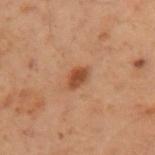Notes:
* biopsy status: imaged on a skin check; not biopsied
* image: ~15 mm tile from a whole-body skin photo
* illumination: cross-polarized illumination
* patient: female, about 55 years old
* anatomic site: the arm
* lesion diameter: ~2.5 mm (longest diameter)
* automated lesion analysis: a lesion area of about 4 mm², a shape eccentricity near 0.65, and two-axis asymmetry of about 0.3